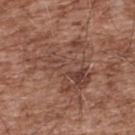The lesion was tiled from a total-body skin photograph and was not biopsied. On the upper back. This is a white-light tile. A region of skin cropped from a whole-body photographic capture, roughly 15 mm wide. The total-body-photography lesion software estimated about 7 CIELAB-L* units darker than the surrounding skin and a normalized border contrast of about 6. The subject is a male aged approximately 55.